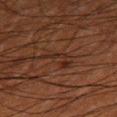| key | value |
|---|---|
| notes | total-body-photography surveillance lesion; no biopsy |
| body site | the right thigh |
| size | about 3.5 mm |
| imaging modality | ~15 mm tile from a whole-body skin photo |
| subject | male, aged approximately 50 |